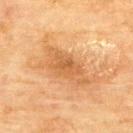| feature | finding |
|---|---|
| workup | catalogued during a skin exam; not biopsied |
| image-analysis metrics | a lesion area of about 12 mm², a shape eccentricity near 0.9, and a shape-asymmetry score of about 0.35 (0 = symmetric); about 8 CIELAB-L* units darker than the surrounding skin; a border-irregularity index near 4.5/10, internal color variation of about 3 on a 0–10 scale, and radial color variation of about 1; a nevus-likeness score of about 0/100 |
| patient | female, aged 78 to 82 |
| lesion size | ~6 mm (longest diameter) |
| body site | the back |
| imaging modality | total-body-photography crop, ~15 mm field of view |
| lighting | cross-polarized illumination |